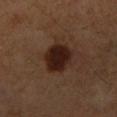notes: total-body-photography surveillance lesion; no biopsy | image-analysis metrics: a footprint of about 11 mm², an outline eccentricity of about 0.55 (0 = round, 1 = elongated), and two-axis asymmetry of about 0.15; a border-irregularity rating of about 1.5/10, a color-variation rating of about 2.5/10, and a peripheral color-asymmetry measure near 0.5; an automated nevus-likeness rating near 85 out of 100 | patient: male, roughly 60 years of age | location: the right lower leg | image source: ~15 mm crop, total-body skin-cancer survey | lesion size: ≈4 mm | tile lighting: cross-polarized.